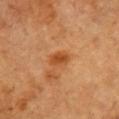Cropped from a total-body skin-imaging series; the visible field is about 15 mm.
Automated tile analysis of the lesion measured a lesion area of about 4.5 mm² and a symmetry-axis asymmetry near 0.2. The software also gave border irregularity of about 2 on a 0–10 scale, a color-variation rating of about 2.5/10, and radial color variation of about 1. The analysis additionally found a classifier nevus-likeness of about 55/100 and lesion-presence confidence of about 100/100.
Imaged with cross-polarized lighting.
The recorded lesion diameter is about 3 mm.
On the chest.
A male patient, in their mid- to late 80s or older.